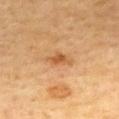On the back. A female subject, approximately 55 years of age. A close-up tile cropped from a whole-body skin photograph, about 15 mm across.